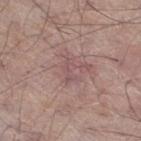Cropped from a total-body skin-imaging series; the visible field is about 15 mm.
Approximately 3 mm at its widest.
Automated image analysis of the tile measured about 6 CIELAB-L* units darker than the surrounding skin. It also reported a lesion-detection confidence of about 90/100.
This is a white-light tile.
A male subject aged around 55.
The lesion is on the right thigh.